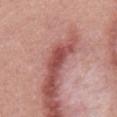workup: catalogued during a skin exam; not biopsied
patient: male, roughly 55 years of age
size: about 3 mm
image: ~15 mm tile from a whole-body skin photo
automated metrics: an outline eccentricity of about 0.9 (0 = round, 1 = elongated) and two-axis asymmetry of about 0.25; an average lesion color of about L≈46 a*≈30 b*≈25 (CIELAB), a lesion–skin lightness drop of about 13, and a normalized border contrast of about 9
anatomic site: the mid back
illumination: white-light illumination